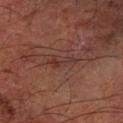The lesion is located on the left forearm.
This is a cross-polarized tile.
A 15 mm crop from a total-body photograph taken for skin-cancer surveillance.
Automated tile analysis of the lesion measured an eccentricity of roughly 0.95 and two-axis asymmetry of about 0.5. The analysis additionally found a border-irregularity rating of about 6/10 and internal color variation of about 1.5 on a 0–10 scale.
About 3.5 mm across.
A male subject, aged approximately 60.